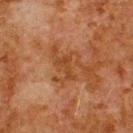Q: Was a biopsy performed?
A: total-body-photography surveillance lesion; no biopsy
Q: Lesion location?
A: the upper back
Q: Who is the patient?
A: male, aged 78–82
Q: What is the imaging modality?
A: ~15 mm crop, total-body skin-cancer survey